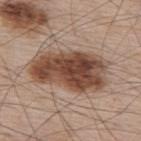Q: Was this lesion biopsied?
A: catalogued during a skin exam; not biopsied
Q: Patient demographics?
A: male, about 65 years old
Q: Illumination type?
A: white-light
Q: Automated lesion metrics?
A: a lesion area of about 32 mm², an eccentricity of roughly 0.85, and a symmetry-axis asymmetry near 0.2; a border-irregularity index near 2.5/10, internal color variation of about 7.5 on a 0–10 scale, and radial color variation of about 2.5; a detector confidence of about 100 out of 100 that the crop contains a lesion
Q: Lesion size?
A: about 8.5 mm
Q: Lesion location?
A: the upper back
Q: What kind of image is this?
A: ~15 mm crop, total-body skin-cancer survey